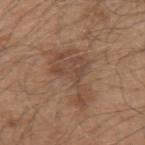Q: Is there a histopathology result?
A: total-body-photography surveillance lesion; no biopsy
Q: Patient demographics?
A: male, approximately 50 years of age
Q: Automated lesion metrics?
A: an area of roughly 13 mm², an outline eccentricity of about 0.85 (0 = round, 1 = elongated), and two-axis asymmetry of about 0.7; roughly 8 lightness units darker than nearby skin and a normalized lesion–skin contrast near 6.5
Q: How was the tile lit?
A: white-light illumination
Q: What kind of image is this?
A: ~15 mm crop, total-body skin-cancer survey
Q: Lesion location?
A: the arm
Q: Lesion size?
A: about 6 mm